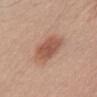Notes:
• workup — catalogued during a skin exam; not biopsied
• illumination — white-light
• patient — male, in their mid- to late 60s
• diameter — ~4.5 mm (longest diameter)
• image source — total-body-photography crop, ~15 mm field of view
• automated metrics — an average lesion color of about L≈54 a*≈22 b*≈29 (CIELAB), about 11 CIELAB-L* units darker than the surrounding skin, and a lesion-to-skin contrast of about 7.5 (normalized; higher = more distinct); a border-irregularity index near 2/10 and a within-lesion color-variation index near 3/10
• body site — the abdomen